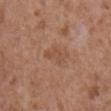{"biopsy_status": "not biopsied; imaged during a skin examination", "image": {"source": "total-body photography crop", "field_of_view_mm": 15}, "site": "abdomen", "patient": {"sex": "male", "age_approx": 75}, "lighting": "white-light"}